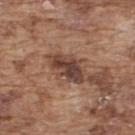Clinical impression:
The lesion was tiled from a total-body skin photograph and was not biopsied.
Context:
A 15 mm close-up tile from a total-body photography series done for melanoma screening. The lesion is on the upper back. A male patient, aged around 75. Automated tile analysis of the lesion measured an eccentricity of roughly 0.85 and two-axis asymmetry of about 0.25. It also reported a lesion color around L≈41 a*≈19 b*≈25 in CIELAB, roughly 13 lightness units darker than nearby skin, and a lesion-to-skin contrast of about 10.5 (normalized; higher = more distinct). And it measured border irregularity of about 3 on a 0–10 scale, a color-variation rating of about 5.5/10, and a peripheral color-asymmetry measure near 2.